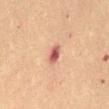Image and clinical context: Automated image analysis of the tile measured a footprint of about 4 mm². And it measured a lesion color around L≈51 a*≈25 b*≈26 in CIELAB and a normalized border contrast of about 9.5. The analysis additionally found internal color variation of about 3 on a 0–10 scale and a peripheral color-asymmetry measure near 0.5. The software also gave a nevus-likeness score of about 0/100 and lesion-presence confidence of about 100/100. On the front of the torso. The tile uses cross-polarized illumination. The subject is a female roughly 70 years of age. A lesion tile, about 15 mm wide, cut from a 3D total-body photograph.